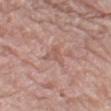<tbp_lesion>
  <site>left thigh</site>
  <automated_metrics>
    <area_mm2_approx>4.0</area_mm2_approx>
    <eccentricity>0.75</eccentricity>
    <shape_asymmetry>0.6</shape_asymmetry>
    <cielab_L>56</cielab_L>
    <cielab_a>21</cielab_a>
    <cielab_b>25</cielab_b>
    <vs_skin_darker_L>7.0</vs_skin_darker_L>
    <vs_skin_contrast_norm>5.0</vs_skin_contrast_norm>
    <border_irregularity_0_10>6.0</border_irregularity_0_10>
    <color_variation_0_10>1.0</color_variation_0_10>
    <peripheral_color_asymmetry>0.5</peripheral_color_asymmetry>
    <nevus_likeness_0_100>0</nevus_likeness_0_100>
  </automated_metrics>
  <patient>
    <sex>female</sex>
    <age_approx>60</age_approx>
  </patient>
  <image>
    <source>total-body photography crop</source>
    <field_of_view_mm>15</field_of_view_mm>
  </image>
  <lesion_size>
    <long_diameter_mm_approx>3.0</long_diameter_mm_approx>
  </lesion_size>
</tbp_lesion>